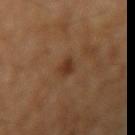<tbp_lesion>
  <biopsy_status>not biopsied; imaged during a skin examination</biopsy_status>
  <patient>
    <sex>male</sex>
    <age_approx>55</age_approx>
  </patient>
  <lighting>cross-polarized</lighting>
  <image>
    <source>total-body photography crop</source>
    <field_of_view_mm>15</field_of_view_mm>
  </image>
  <lesion_size>
    <long_diameter_mm_approx>2.5</long_diameter_mm_approx>
  </lesion_size>
  <site>right upper arm</site>
</tbp_lesion>